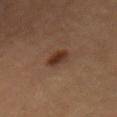This lesion was catalogued during total-body skin photography and was not selected for biopsy.
A roughly 15 mm field-of-view crop from a total-body skin photograph.
About 3 mm across.
The subject is a female roughly 60 years of age.
The lesion is on the front of the torso.